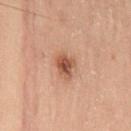size: ≈2.5 mm | subject: male, aged approximately 60 | site: the back | acquisition: ~15 mm crop, total-body skin-cancer survey | tile lighting: cross-polarized | TBP lesion metrics: border irregularity of about 2.5 on a 0–10 scale, internal color variation of about 3.5 on a 0–10 scale, and peripheral color asymmetry of about 1.